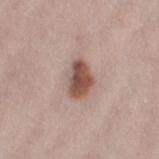biopsy status — catalogued during a skin exam; not biopsied | acquisition — 15 mm crop, total-body photography | patient — female, roughly 40 years of age | body site — the left thigh.